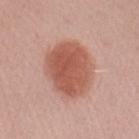Clinical impression: No biopsy was performed on this lesion — it was imaged during a full skin examination and was not determined to be concerning. Image and clinical context: Measured at roughly 6.5 mm in maximum diameter. A 15 mm close-up tile from a total-body photography series done for melanoma screening. A female subject roughly 60 years of age. Located on the left forearm.